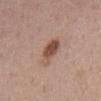workup = catalogued during a skin exam; not biopsied
patient = male, in their 60s
lesion size = ≈4.5 mm
illumination = white-light illumination
anatomic site = the mid back
imaging modality = 15 mm crop, total-body photography